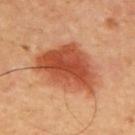Clinical impression: Part of a total-body skin-imaging series; this lesion was reviewed on a skin check and was not flagged for biopsy. Clinical summary: A male patient, aged 63 to 67. From the upper back. A 15 mm close-up tile from a total-body photography series done for melanoma screening.